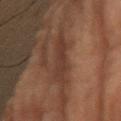No biopsy was performed on this lesion — it was imaged during a full skin examination and was not determined to be concerning.